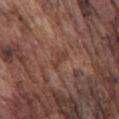biopsy status — imaged on a skin check; not biopsied
body site — the front of the torso
patient — male, in their mid-70s
acquisition — total-body-photography crop, ~15 mm field of view